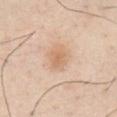follow-up: total-body-photography surveillance lesion; no biopsy
tile lighting: white-light
automated lesion analysis: an area of roughly 5 mm², an eccentricity of roughly 0.6, and a shape-asymmetry score of about 0.2 (0 = symmetric); a lesion color around L≈67 a*≈19 b*≈34 in CIELAB, about 8 CIELAB-L* units darker than the surrounding skin, and a normalized lesion–skin contrast near 6; a border-irregularity rating of about 1.5/10, a color-variation rating of about 2/10, and peripheral color asymmetry of about 0.5; a nevus-likeness score of about 20/100 and lesion-presence confidence of about 100/100
image source: total-body-photography crop, ~15 mm field of view
anatomic site: the chest
subject: male, aged around 30